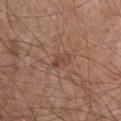Recorded during total-body skin imaging; not selected for excision or biopsy. This is a white-light tile. About 3 mm across. On the front of the torso. Cropped from a whole-body photographic skin survey; the tile spans about 15 mm. A male subject roughly 65 years of age.